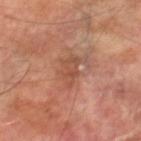Impression:
This lesion was catalogued during total-body skin photography and was not selected for biopsy.
Image and clinical context:
A roughly 15 mm field-of-view crop from a total-body skin photograph. The lesion is located on the leg. A male subject, about 70 years old.